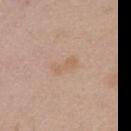<tbp_lesion>
  <biopsy_status>not biopsied; imaged during a skin examination</biopsy_status>
  <patient>
    <sex>female</sex>
    <age_approx>70</age_approx>
  </patient>
  <lighting>white-light</lighting>
  <automated_metrics>
    <shape_asymmetry>0.3</shape_asymmetry>
    <cielab_L>61</cielab_L>
    <cielab_a>17</cielab_a>
    <cielab_b>31</cielab_b>
    <vs_skin_darker_L>6.0</vs_skin_darker_L>
    <vs_skin_contrast_norm>5.0</vs_skin_contrast_norm>
    <peripheral_color_asymmetry>0.0</peripheral_color_asymmetry>
    <lesion_detection_confidence_0_100>100</lesion_detection_confidence_0_100>
  </automated_metrics>
  <image>
    <source>total-body photography crop</source>
    <field_of_view_mm>15</field_of_view_mm>
  </image>
  <site>chest</site>
</tbp_lesion>